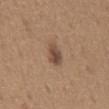workup: no biopsy performed (imaged during a skin exam) | patient: male, aged 63 to 67 | acquisition: total-body-photography crop, ~15 mm field of view | automated lesion analysis: a shape eccentricity near 0.8 and a shape-asymmetry score of about 0.3 (0 = symmetric); a lesion–skin lightness drop of about 11 and a lesion-to-skin contrast of about 8 (normalized; higher = more distinct) | illumination: white-light | body site: the mid back.